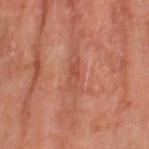No biopsy was performed on this lesion — it was imaged during a full skin examination and was not determined to be concerning.
Approximately 3 mm at its widest.
Automated tile analysis of the lesion measured a lesion color around L≈48 a*≈28 b*≈30 in CIELAB, roughly 7 lightness units darker than nearby skin, and a lesion-to-skin contrast of about 5.5 (normalized; higher = more distinct).
The lesion is on the left thigh.
The subject is a female aged 68–72.
A 15 mm crop from a total-body photograph taken for skin-cancer surveillance.
Imaged with cross-polarized lighting.